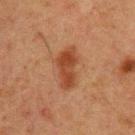{"biopsy_status": "not biopsied; imaged during a skin examination", "lighting": "cross-polarized", "patient": {"sex": "male", "age_approx": 60}, "site": "back", "image": {"source": "total-body photography crop", "field_of_view_mm": 15}, "lesion_size": {"long_diameter_mm_approx": 5.0}}